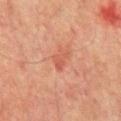<lesion>
<biopsy_status>not biopsied; imaged during a skin examination</biopsy_status>
<site>chest</site>
<image>
  <source>total-body photography crop</source>
  <field_of_view_mm>15</field_of_view_mm>
</image>
<patient>
  <sex>male</sex>
  <age_approx>75</age_approx>
</patient>
</lesion>